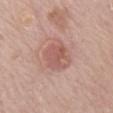Imaged during a routine full-body skin examination; the lesion was not biopsied and no histopathology is available. Automated tile analysis of the lesion measured a lesion area of about 9 mm², an eccentricity of roughly 0.65, and a shape-asymmetry score of about 0.2 (0 = symmetric). The software also gave an automated nevus-likeness rating near 35 out of 100. About 4 mm across. The subject is a female in their mid-80s or older. Imaged with white-light lighting. Located on the back. This image is a 15 mm lesion crop taken from a total-body photograph.